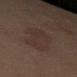<record>
<biopsy_status>not biopsied; imaged during a skin examination</biopsy_status>
<site>right thigh</site>
<lesion_size>
  <long_diameter_mm_approx>6.0</long_diameter_mm_approx>
</lesion_size>
<image>
  <source>total-body photography crop</source>
  <field_of_view_mm>15</field_of_view_mm>
</image>
<patient>
  <sex>female</sex>
  <age_approx>55</age_approx>
</patient>
<lighting>cross-polarized</lighting>
</record>